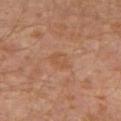• follow-up · no biopsy performed (imaged during a skin exam)
• tile lighting · cross-polarized illumination
• size · about 3 mm
• patient · male, in their 30s
• site · the left leg
• automated lesion analysis · an area of roughly 4.5 mm², an eccentricity of roughly 0.75, and a shape-asymmetry score of about 0.25 (0 = symmetric); border irregularity of about 2.5 on a 0–10 scale, a within-lesion color-variation index near 1.5/10, and peripheral color asymmetry of about 0.5
• imaging modality · ~15 mm tile from a whole-body skin photo